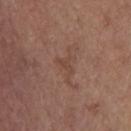No biopsy was performed on this lesion — it was imaged during a full skin examination and was not determined to be concerning.
From the chest.
Measured at roughly 2.5 mm in maximum diameter.
Automated image analysis of the tile measured an area of roughly 2 mm² and a shape-asymmetry score of about 0.45 (0 = symmetric). The software also gave a mean CIELAB color near L≈44 a*≈19 b*≈26, a lesion–skin lightness drop of about 5, and a normalized border contrast of about 4.5. And it measured a border-irregularity index near 5/10, a within-lesion color-variation index near 0/10, and a peripheral color-asymmetry measure near 0.
A 15 mm crop from a total-body photograph taken for skin-cancer surveillance.
A female patient in their mid-60s.
This is a white-light tile.